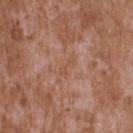Q: Was this lesion biopsied?
A: catalogued during a skin exam; not biopsied
Q: Who is the patient?
A: male, roughly 45 years of age
Q: How was this image acquired?
A: ~15 mm tile from a whole-body skin photo
Q: What did automated image analysis measure?
A: a footprint of about 3 mm² and a shape eccentricity near 0.9; roughly 5 lightness units darker than nearby skin and a normalized border contrast of about 5; border irregularity of about 4.5 on a 0–10 scale and a peripheral color-asymmetry measure near 0
Q: What lighting was used for the tile?
A: white-light illumination
Q: Lesion size?
A: ≈3 mm
Q: What is the anatomic site?
A: the upper back